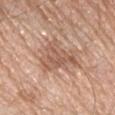notes: no biopsy performed (imaged during a skin exam)
imaging modality: 15 mm crop, total-body photography
subject: male, aged approximately 70
illumination: white-light
site: the left forearm
lesion size: about 5 mm